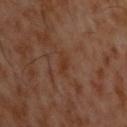• body site — the upper back
• image — ~15 mm crop, total-body skin-cancer survey
• patient — male, roughly 60 years of age